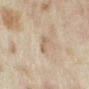The lesion was tiled from a total-body skin photograph and was not biopsied. The lesion is located on the arm. The recorded lesion diameter is about 3 mm. Automated tile analysis of the lesion measured a nevus-likeness score of about 0/100 and lesion-presence confidence of about 60/100. The patient is a female about 35 years old. A 15 mm crop from a total-body photograph taken for skin-cancer surveillance.